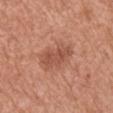Q: Was a biopsy performed?
A: no biopsy performed (imaged during a skin exam)
Q: What is the imaging modality?
A: ~15 mm tile from a whole-body skin photo
Q: What lighting was used for the tile?
A: white-light
Q: Automated lesion metrics?
A: a lesion area of about 9 mm², a shape eccentricity near 0.8, and a symmetry-axis asymmetry near 0.25; border irregularity of about 3.5 on a 0–10 scale, internal color variation of about 2.5 on a 0–10 scale, and peripheral color asymmetry of about 1; a classifier nevus-likeness of about 35/100 and a detector confidence of about 100 out of 100 that the crop contains a lesion
Q: What is the lesion's diameter?
A: ≈4.5 mm
Q: What are the patient's age and sex?
A: male, aged approximately 45
Q: Lesion location?
A: the right upper arm